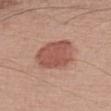This lesion was catalogued during total-body skin photography and was not selected for biopsy. A close-up tile cropped from a whole-body skin photograph, about 15 mm across. Captured under white-light illumination. The patient is a male aged 58 to 62. The recorded lesion diameter is about 4.5 mm. Located on the arm. The lesion-visualizer software estimated an average lesion color of about L≈53 a*≈24 b*≈27 (CIELAB), about 11 CIELAB-L* units darker than the surrounding skin, and a lesion-to-skin contrast of about 7.5 (normalized; higher = more distinct). It also reported an automated nevus-likeness rating near 100 out of 100 and lesion-presence confidence of about 100/100.